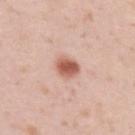No biopsy was performed on this lesion — it was imaged during a full skin examination and was not determined to be concerning. On the upper back. This is a white-light tile. The lesion's longest dimension is about 3 mm. A close-up tile cropped from a whole-body skin photograph, about 15 mm across. A male subject about 35 years old.